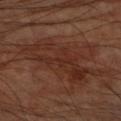notes: total-body-photography surveillance lesion; no biopsy | imaging modality: ~15 mm crop, total-body skin-cancer survey | anatomic site: the left upper arm | subject: male, aged 58 to 62.